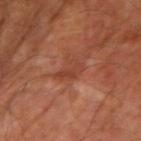notes = total-body-photography surveillance lesion; no biopsy
acquisition = ~15 mm tile from a whole-body skin photo
subject = male, aged approximately 70
location = the right upper arm